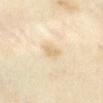Recorded during total-body skin imaging; not selected for excision or biopsy.
The lesion is on the abdomen.
The patient is a female aged approximately 55.
A roughly 15 mm field-of-view crop from a total-body skin photograph.